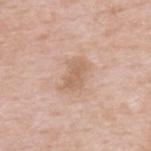| feature | finding |
|---|---|
| workup | imaged on a skin check; not biopsied |
| location | the upper back |
| subject | male, approximately 50 years of age |
| image | ~15 mm crop, total-body skin-cancer survey |